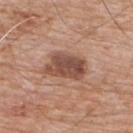{
  "biopsy_status": "not biopsied; imaged during a skin examination",
  "patient": {
    "sex": "male",
    "age_approx": 80
  },
  "automated_metrics": {
    "cielab_L": 49,
    "cielab_a": 21,
    "cielab_b": 28,
    "vs_skin_darker_L": 13.0,
    "border_irregularity_0_10": 2.5,
    "color_variation_0_10": 4.5
  },
  "lighting": "white-light",
  "site": "back",
  "lesion_size": {
    "long_diameter_mm_approx": 4.5
  },
  "image": {
    "source": "total-body photography crop",
    "field_of_view_mm": 15
  }
}